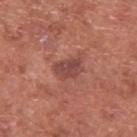An algorithmic analysis of the crop reported a mean CIELAB color near L≈45 a*≈25 b*≈25 and a lesion–skin lightness drop of about 9. And it measured border irregularity of about 2.5 on a 0–10 scale and internal color variation of about 2.5 on a 0–10 scale. The analysis additionally found a nevus-likeness score of about 0/100 and lesion-presence confidence of about 100/100.
Longest diameter approximately 3.5 mm.
Imaged with white-light lighting.
A male patient, aged approximately 75.
A close-up tile cropped from a whole-body skin photograph, about 15 mm across.
From the upper back.